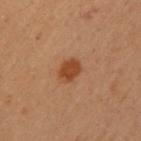{"biopsy_status": "not biopsied; imaged during a skin examination", "image": {"source": "total-body photography crop", "field_of_view_mm": 15}, "patient": {"sex": "female", "age_approx": 40}, "lesion_size": {"long_diameter_mm_approx": 2.5}, "site": "left upper arm"}